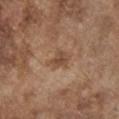The lesion was photographed on a routine skin check and not biopsied; there is no pathology result. The subject is a male about 75 years old. A lesion tile, about 15 mm wide, cut from a 3D total-body photograph. The lesion is located on the chest.